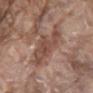workup: no biopsy performed (imaged during a skin exam) | image: ~15 mm crop, total-body skin-cancer survey | location: the back | subject: male, aged around 80.